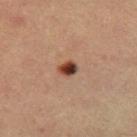Findings:
* biopsy status · no biopsy performed (imaged during a skin exam)
* body site · the right thigh
* imaging modality · ~15 mm tile from a whole-body skin photo
* patient · male, in their mid- to late 60s